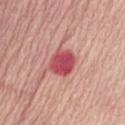The lesion was photographed on a routine skin check and not biopsied; there is no pathology result. On the abdomen. Cropped from a whole-body photographic skin survey; the tile spans about 15 mm. The tile uses white-light illumination. An algorithmic analysis of the crop reported a lesion color around L≈52 a*≈37 b*≈22 in CIELAB, about 15 CIELAB-L* units darker than the surrounding skin, and a lesion-to-skin contrast of about 10 (normalized; higher = more distinct). A female patient in their mid- to late 70s.